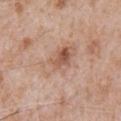Acquisition and patient details: The patient is a male in their mid- to late 60s. An algorithmic analysis of the crop reported a border-irregularity index near 3.5/10, a color-variation rating of about 9/10, and radial color variation of about 3.5. A close-up tile cropped from a whole-body skin photograph, about 15 mm across. Located on the chest. Measured at roughly 6 mm in maximum diameter.